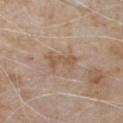{
  "biopsy_status": "not biopsied; imaged during a skin examination",
  "image": {
    "source": "total-body photography crop",
    "field_of_view_mm": 15
  },
  "lesion_size": {
    "long_diameter_mm_approx": 4.0
  },
  "automated_metrics": {
    "border_irregularity_0_10": 4.5,
    "color_variation_0_10": 1.5
  },
  "patient": {
    "sex": "male",
    "age_approx": 80
  },
  "lighting": "white-light",
  "site": "chest"
}